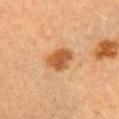Clinical impression:
The lesion was tiled from a total-body skin photograph and was not biopsied.
Image and clinical context:
A close-up tile cropped from a whole-body skin photograph, about 15 mm across. A female subject about 55 years old. The tile uses cross-polarized illumination. From the arm. The lesion's longest dimension is about 3.5 mm.